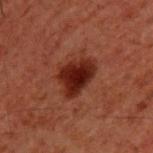{
  "biopsy_status": "not biopsied; imaged during a skin examination",
  "image": {
    "source": "total-body photography crop",
    "field_of_view_mm": 15
  },
  "patient": {
    "sex": "male",
    "age_approx": 60
  },
  "lesion_size": {
    "long_diameter_mm_approx": 4.5
  },
  "site": "upper back"
}